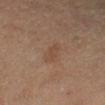{"biopsy_status": "not biopsied; imaged during a skin examination", "patient": {"sex": "female", "age_approx": 55}, "site": "right lower leg", "image": {"source": "total-body photography crop", "field_of_view_mm": 15}}